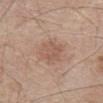Imaged during a routine full-body skin examination; the lesion was not biopsied and no histopathology is available.